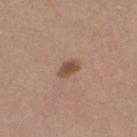Case summary:
• biopsy status — total-body-photography surveillance lesion; no biopsy
• patient — female, aged around 30
• image — ~15 mm tile from a whole-body skin photo
• anatomic site — the leg